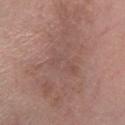Acquisition and patient details: From the left lower leg. A male patient, approximately 25 years of age. A region of skin cropped from a whole-body photographic capture, roughly 15 mm wide. The recorded lesion diameter is about 13.5 mm. The tile uses white-light illumination.